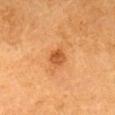subject — female, roughly 65 years of age | tile lighting — cross-polarized | site — the head or neck | size — ≈2 mm | imaging modality — 15 mm crop, total-body photography.